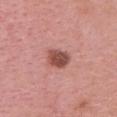The lesion was photographed on a routine skin check and not biopsied; there is no pathology result. The recorded lesion diameter is about 3 mm. Located on the head or neck. A close-up tile cropped from a whole-body skin photograph, about 15 mm across. The subject is a female aged 48–52. Captured under white-light illumination. Automated image analysis of the tile measured a lesion area of about 6 mm², a shape eccentricity near 0.65, and two-axis asymmetry of about 0.25. And it measured a lesion color around L≈50 a*≈26 b*≈26 in CIELAB, about 14 CIELAB-L* units darker than the surrounding skin, and a normalized border contrast of about 9.5. The analysis additionally found border irregularity of about 2 on a 0–10 scale, a within-lesion color-variation index near 3.5/10, and radial color variation of about 1.